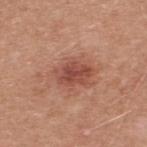Recorded during total-body skin imaging; not selected for excision or biopsy.
This is a white-light tile.
Located on the back.
The patient is a male aged 48–52.
A 15 mm close-up extracted from a 3D total-body photography capture.